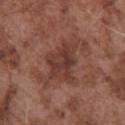  image:
    source: total-body photography crop
    field_of_view_mm: 15
  site: chest
  patient:
    sex: male
    age_approx: 75
  lesion_size:
    long_diameter_mm_approx: 4.5
  lighting: white-light